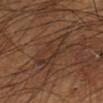workup: imaged on a skin check; not biopsied
imaging modality: total-body-photography crop, ~15 mm field of view
site: the left lower leg
tile lighting: cross-polarized
lesion diameter: ≈6 mm
patient: male, about 55 years old